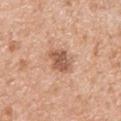location: the right upper arm
TBP lesion metrics: a shape-asymmetry score of about 0.2 (0 = symmetric); a classifier nevus-likeness of about 40/100 and a detector confidence of about 100 out of 100 that the crop contains a lesion
tile lighting: white-light
patient: female, about 70 years old
acquisition: total-body-photography crop, ~15 mm field of view